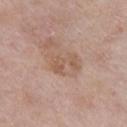Assessment:
The lesion was photographed on a routine skin check and not biopsied; there is no pathology result.
Context:
On the chest. Measured at roughly 3.5 mm in maximum diameter. Captured under white-light illumination. A 15 mm close-up tile from a total-body photography series done for melanoma screening. The lesion-visualizer software estimated an area of roughly 7 mm² and a shape-asymmetry score of about 0.5 (0 = symmetric). The analysis additionally found a lesion color around L≈56 a*≈18 b*≈28 in CIELAB and a normalized lesion–skin contrast near 5.5. The software also gave a within-lesion color-variation index near 2/10 and peripheral color asymmetry of about 0.5. It also reported a nevus-likeness score of about 5/100. The subject is a female aged around 60.